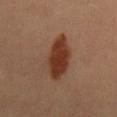Clinical impression:
This lesion was catalogued during total-body skin photography and was not selected for biopsy.
Context:
The recorded lesion diameter is about 6.5 mm. A female subject, aged around 50. A 15 mm crop from a total-body photograph taken for skin-cancer surveillance. Captured under cross-polarized illumination. Automated tile analysis of the lesion measured internal color variation of about 3 on a 0–10 scale and peripheral color asymmetry of about 1. And it measured a detector confidence of about 100 out of 100 that the crop contains a lesion.